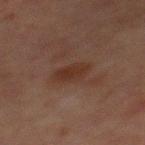Captured during whole-body skin photography for melanoma surveillance; the lesion was not biopsied.
About 3.5 mm across.
This is a cross-polarized tile.
A 15 mm crop from a total-body photograph taken for skin-cancer surveillance.
The patient is a male aged 63 to 67.
The lesion is on the chest.